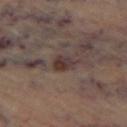Clinical summary:
From the leg. A subject aged 58–62. About 3 mm across. Captured under cross-polarized illumination. A close-up tile cropped from a whole-body skin photograph, about 15 mm across.